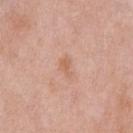workup=catalogued during a skin exam; not biopsied
image source=~15 mm tile from a whole-body skin photo
image-analysis metrics=a peripheral color-asymmetry measure near 0.5
illumination=white-light
location=the chest
patient=male, aged 53 to 57
lesion size=~2.5 mm (longest diameter)